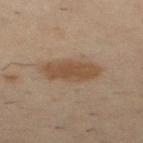The lesion was tiled from a total-body skin photograph and was not biopsied.
About 5.5 mm across.
A region of skin cropped from a whole-body photographic capture, roughly 15 mm wide.
The lesion-visualizer software estimated a lesion color around L≈47 a*≈16 b*≈30 in CIELAB, roughly 9 lightness units darker than nearby skin, and a normalized border contrast of about 8.
Imaged with cross-polarized lighting.
The lesion is located on the upper back.
A male subject aged 38–42.